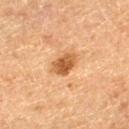This lesion was catalogued during total-body skin photography and was not selected for biopsy. The total-body-photography lesion software estimated an area of roughly 5.5 mm² and an eccentricity of roughly 0.65. And it measured a lesion color around L≈45 a*≈20 b*≈35 in CIELAB, about 12 CIELAB-L* units darker than the surrounding skin, and a normalized lesion–skin contrast near 9.5. The software also gave a border-irregularity index near 2/10, internal color variation of about 3.5 on a 0–10 scale, and radial color variation of about 1. And it measured a nevus-likeness score of about 80/100 and lesion-presence confidence of about 100/100. A male patient, aged approximately 75. The lesion's longest dimension is about 3 mm. On the left thigh. The tile uses cross-polarized illumination. A 15 mm crop from a total-body photograph taken for skin-cancer surveillance.